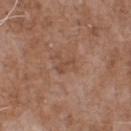follow-up = no biopsy performed (imaged during a skin exam) | subject = male, aged 68–72 | acquisition = ~15 mm crop, total-body skin-cancer survey | automated metrics = border irregularity of about 4 on a 0–10 scale, a within-lesion color-variation index near 0.5/10, and peripheral color asymmetry of about 0; a classifier nevus-likeness of about 0/100 and lesion-presence confidence of about 100/100 | site = the chest.